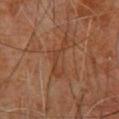Assessment: Part of a total-body skin-imaging series; this lesion was reviewed on a skin check and was not flagged for biopsy. Acquisition and patient details: An algorithmic analysis of the crop reported a mean CIELAB color near L≈36 a*≈20 b*≈29, a lesion–skin lightness drop of about 6, and a normalized lesion–skin contrast near 5.5. A male subject, in their 60s. Captured under cross-polarized illumination. Located on the upper back. Cropped from a whole-body photographic skin survey; the tile spans about 15 mm.